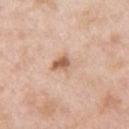Q: Was a biopsy performed?
A: catalogued during a skin exam; not biopsied